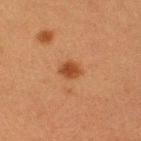Case summary:
– notes: imaged on a skin check; not biopsied
– acquisition: 15 mm crop, total-body photography
– anatomic site: the leg
– patient: female, aged 38 to 42